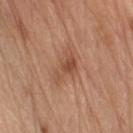Impression: Recorded during total-body skin imaging; not selected for excision or biopsy. Context: A 15 mm close-up tile from a total-body photography series done for melanoma screening. From the left upper arm. The tile uses white-light illumination. A male patient roughly 70 years of age.